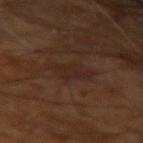Q: Is there a histopathology result?
A: total-body-photography surveillance lesion; no biopsy
Q: What kind of image is this?
A: total-body-photography crop, ~15 mm field of view
Q: Where on the body is the lesion?
A: the left arm
Q: What did automated image analysis measure?
A: an area of roughly 6.5 mm², an eccentricity of roughly 0.8, and a shape-asymmetry score of about 0.2 (0 = symmetric); a lesion–skin lightness drop of about 4 and a normalized lesion–skin contrast near 5.5; a classifier nevus-likeness of about 0/100 and a detector confidence of about 95 out of 100 that the crop contains a lesion
Q: How was the tile lit?
A: cross-polarized
Q: What are the patient's age and sex?
A: male, aged around 60
Q: Lesion size?
A: about 3.5 mm